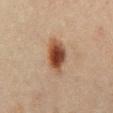biopsy_status: not biopsied; imaged during a skin examination
image:
  source: total-body photography crop
  field_of_view_mm: 15
patient:
  sex: male
  age_approx: 45
lighting: cross-polarized
lesion_size:
  long_diameter_mm_approx: 4.5
automated_metrics:
  area_mm2_approx: 9.5
  eccentricity: 0.8
  shape_asymmetry: 0.2
  vs_skin_darker_L: 13.0
  vs_skin_contrast_norm: 11.5
  nevus_likeness_0_100: 100
  lesion_detection_confidence_0_100: 100
site: abdomen